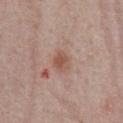Notes:
– follow-up · imaged on a skin check; not biopsied
– imaging modality · 15 mm crop, total-body photography
– body site · the chest
– lesion size · about 2.5 mm
– illumination · white-light illumination
– patient · male, in their mid- to late 50s